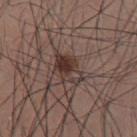Imaged during a routine full-body skin examination; the lesion was not biopsied and no histopathology is available. Longest diameter approximately 4 mm. The tile uses white-light illumination. On the front of the torso. A male patient aged approximately 35. A 15 mm close-up tile from a total-body photography series done for melanoma screening. The total-body-photography lesion software estimated an eccentricity of roughly 0.75 and a shape-asymmetry score of about 0.25 (0 = symmetric). The analysis additionally found a lesion color around L≈35 a*≈16 b*≈20 in CIELAB, about 10 CIELAB-L* units darker than the surrounding skin, and a lesion-to-skin contrast of about 8.5 (normalized; higher = more distinct). It also reported a color-variation rating of about 8.5/10 and radial color variation of about 3.5. And it measured an automated nevus-likeness rating near 100 out of 100 and a detector confidence of about 100 out of 100 that the crop contains a lesion.